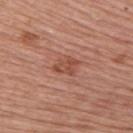Q: Is there a histopathology result?
A: no biopsy performed (imaged during a skin exam)
Q: How was this image acquired?
A: ~15 mm tile from a whole-body skin photo
Q: What did automated image analysis measure?
A: a lesion area of about 4.5 mm², a shape eccentricity near 0.75, and a shape-asymmetry score of about 0.3 (0 = symmetric); an average lesion color of about L≈49 a*≈26 b*≈29 (CIELAB), a lesion–skin lightness drop of about 9, and a lesion-to-skin contrast of about 6.5 (normalized; higher = more distinct); an automated nevus-likeness rating near 0 out of 100 and a lesion-detection confidence of about 100/100
Q: Lesion location?
A: the right upper arm
Q: Who is the patient?
A: female, aged approximately 65
Q: Lesion size?
A: about 3 mm